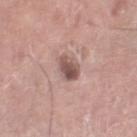Clinical impression: This lesion was catalogued during total-body skin photography and was not selected for biopsy. Image and clinical context: A 15 mm close-up extracted from a 3D total-body photography capture. On the right lower leg. About 3.5 mm across. A male patient in their mid-70s. The tile uses white-light illumination.